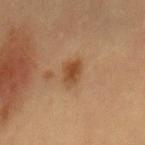acquisition: ~15 mm crop, total-body skin-cancer survey
site: the back
lesion size: about 3 mm
tile lighting: cross-polarized
patient: female, aged around 60